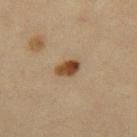<lesion>
  <biopsy_status>not biopsied; imaged during a skin examination</biopsy_status>
  <automated_metrics>
    <cielab_L>38</cielab_L>
    <cielab_a>16</cielab_a>
    <cielab_b>30</cielab_b>
    <vs_skin_darker_L>13.0</vs_skin_darker_L>
    <vs_skin_contrast_norm>11.5</vs_skin_contrast_norm>
    <color_variation_0_10>5.5</color_variation_0_10>
    <peripheral_color_asymmetry>2.0</peripheral_color_asymmetry>
  </automated_metrics>
  <patient>
    <sex>female</sex>
    <age_approx>55</age_approx>
  </patient>
  <lighting>cross-polarized</lighting>
  <site>right thigh</site>
  <image>
    <source>total-body photography crop</source>
    <field_of_view_mm>15</field_of_view_mm>
  </image>
  <lesion_size>
    <long_diameter_mm_approx>3.0</long_diameter_mm_approx>
  </lesion_size>
</lesion>